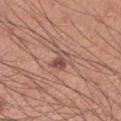Findings:
- workup · imaged on a skin check; not biopsied
- automated lesion analysis · an average lesion color of about L≈51 a*≈21 b*≈25 (CIELAB), a lesion–skin lightness drop of about 9, and a normalized border contrast of about 6.5; a border-irregularity index near 3/10 and a peripheral color-asymmetry measure near 3; a nevus-likeness score of about 20/100
- tile lighting · white-light illumination
- image source · total-body-photography crop, ~15 mm field of view
- subject · male, aged 33 to 37
- diameter · ~3 mm (longest diameter)
- site · the left upper arm